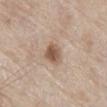• tile lighting: white-light illumination
• automated metrics: an area of roughly 5.5 mm², an outline eccentricity of about 0.55 (0 = round, 1 = elongated), and two-axis asymmetry of about 0.2; a mean CIELAB color near L≈55 a*≈17 b*≈29 and a normalized lesion–skin contrast near 9; border irregularity of about 1.5 on a 0–10 scale and peripheral color asymmetry of about 1
• patient: male, roughly 80 years of age
• image source: ~15 mm tile from a whole-body skin photo
• location: the abdomen